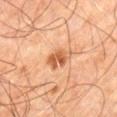Captured during whole-body skin photography for melanoma surveillance; the lesion was not biopsied.
A close-up tile cropped from a whole-body skin photograph, about 15 mm across.
Automated tile analysis of the lesion measured an average lesion color of about L≈58 a*≈26 b*≈39 (CIELAB), roughly 12 lightness units darker than nearby skin, and a lesion-to-skin contrast of about 8 (normalized; higher = more distinct). The analysis additionally found a detector confidence of about 100 out of 100 that the crop contains a lesion.
From the right leg.
The tile uses cross-polarized illumination.
A male subject about 60 years old.